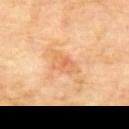<lesion>
<biopsy_status>not biopsied; imaged during a skin examination</biopsy_status>
<lesion_size>
  <long_diameter_mm_approx>4.0</long_diameter_mm_approx>
</lesion_size>
<patient>
  <sex>male</sex>
  <age_approx>70</age_approx>
</patient>
<site>mid back</site>
<image>
  <source>total-body photography crop</source>
  <field_of_view_mm>15</field_of_view_mm>
</image>
<lighting>cross-polarized</lighting>
</lesion>